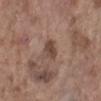{"biopsy_status": "not biopsied; imaged during a skin examination", "lesion_size": {"long_diameter_mm_approx": 2.5}, "site": "left lower leg", "image": {"source": "total-body photography crop", "field_of_view_mm": 15}, "patient": {"sex": "female", "age_approx": "85+"}}